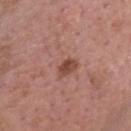workup — catalogued during a skin exam; not biopsied | site — the head or neck | illumination — white-light illumination | subject — male, about 40 years old | image — ~15 mm crop, total-body skin-cancer survey | diameter — ≈5.5 mm.